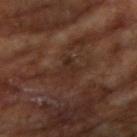Part of a total-body skin-imaging series; this lesion was reviewed on a skin check and was not flagged for biopsy. This image is a 15 mm lesion crop taken from a total-body photograph. The lesion is located on the right upper arm. The subject is a male approximately 65 years of age.